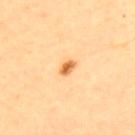Findings:
- workup · catalogued during a skin exam; not biopsied
- subject · male, aged approximately 65
- image source · ~15 mm crop, total-body skin-cancer survey
- image-analysis metrics · a footprint of about 2.5 mm² and a shape eccentricity near 0.8; a border-irregularity rating of about 2/10 and a within-lesion color-variation index near 2/10
- tile lighting · cross-polarized
- size · ≈2 mm
- site · the upper back